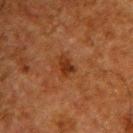Q: Was a biopsy performed?
A: no biopsy performed (imaged during a skin exam)
Q: Lesion location?
A: the upper back
Q: What did automated image analysis measure?
A: an average lesion color of about L≈26 a*≈22 b*≈29 (CIELAB), roughly 8 lightness units darker than nearby skin, and a lesion-to-skin contrast of about 8.5 (normalized; higher = more distinct); an automated nevus-likeness rating near 55 out of 100 and lesion-presence confidence of about 100/100
Q: How was the tile lit?
A: cross-polarized illumination
Q: What is the imaging modality?
A: 15 mm crop, total-body photography
Q: What is the lesion's diameter?
A: ≈2.5 mm
Q: Patient demographics?
A: male, aged approximately 60